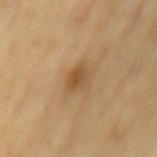biopsy status — total-body-photography surveillance lesion; no biopsy
imaging modality — ~15 mm tile from a whole-body skin photo
lesion size — ≈3.5 mm
anatomic site — the mid back
automated lesion analysis — an area of roughly 6 mm², an eccentricity of roughly 0.65, and a shape-asymmetry score of about 0.2 (0 = symmetric)
patient — male, aged around 70